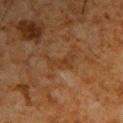<case>
<biopsy_status>not biopsied; imaged during a skin examination</biopsy_status>
<lesion_size>
  <long_diameter_mm_approx>4.0</long_diameter_mm_approx>
</lesion_size>
<patient>
  <sex>male</sex>
  <age_approx>65</age_approx>
</patient>
<automated_metrics>
  <eccentricity>0.9</eccentricity>
  <shape_asymmetry>0.5</shape_asymmetry>
  <cielab_L>30</cielab_L>
  <cielab_a>17</cielab_a>
  <cielab_b>29</cielab_b>
  <vs_skin_contrast_norm>4.5</vs_skin_contrast_norm>
</automated_metrics>
<site>upper back</site>
<lighting>cross-polarized</lighting>
<image>
  <source>total-body photography crop</source>
  <field_of_view_mm>15</field_of_view_mm>
</image>
</case>